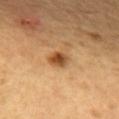biopsy_status: not biopsied; imaged during a skin examination
image:
  source: total-body photography crop
  field_of_view_mm: 15
lighting: cross-polarized
patient:
  sex: female
  age_approx: 40
site: upper back
lesion_size:
  long_diameter_mm_approx: 2.5
automated_metrics:
  area_mm2_approx: 4.0
  shape_asymmetry: 0.2
  cielab_L: 51
  cielab_a: 23
  cielab_b: 40
  nevus_likeness_0_100: 95
  lesion_detection_confidence_0_100: 100